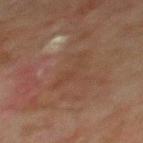Impression:
Recorded during total-body skin imaging; not selected for excision or biopsy.
Image and clinical context:
The tile uses cross-polarized illumination. The lesion's longest dimension is about 4.5 mm. A 15 mm crop from a total-body photograph taken for skin-cancer surveillance. A male patient aged 68–72. On the back.